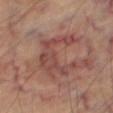Assessment: Captured during whole-body skin photography for melanoma surveillance; the lesion was not biopsied. Background: Measured at roughly 6 mm in maximum diameter. The tile uses cross-polarized illumination. Cropped from a total-body skin-imaging series; the visible field is about 15 mm. A male patient, aged 68 to 72. Located on the right thigh.